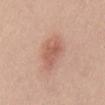Q: Was a biopsy performed?
A: imaged on a skin check; not biopsied
Q: How was this image acquired?
A: 15 mm crop, total-body photography
Q: What lighting was used for the tile?
A: white-light illumination
Q: Automated lesion metrics?
A: an area of roughly 10 mm² and a symmetry-axis asymmetry near 0.25; a lesion color around L≈59 a*≈22 b*≈29 in CIELAB, about 9 CIELAB-L* units darker than the surrounding skin, and a normalized border contrast of about 6.5; a border-irregularity index near 2.5/10, a within-lesion color-variation index near 3/10, and peripheral color asymmetry of about 1
Q: Lesion size?
A: about 5 mm
Q: Who is the patient?
A: male, in their 50s
Q: What is the anatomic site?
A: the abdomen